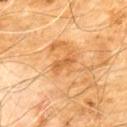The lesion is on the chest. Automated tile analysis of the lesion measured an average lesion color of about L≈59 a*≈25 b*≈46 (CIELAB), roughly 9 lightness units darker than nearby skin, and a lesion-to-skin contrast of about 6 (normalized; higher = more distinct). And it measured a border-irregularity index near 3/10, a within-lesion color-variation index near 1/10, and peripheral color asymmetry of about 0.5. The analysis additionally found a classifier nevus-likeness of about 0/100 and lesion-presence confidence of about 100/100. The recorded lesion diameter is about 3 mm. A 15 mm crop from a total-body photograph taken for skin-cancer surveillance. The subject is a male about 60 years old.